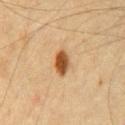The recorded lesion diameter is about 3 mm. The tile uses cross-polarized illumination. A close-up tile cropped from a whole-body skin photograph, about 15 mm across. From the chest. A male subject roughly 65 years of age.